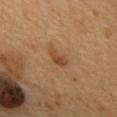follow-up = no biopsy performed (imaged during a skin exam)
illumination = cross-polarized illumination
patient = male, aged 53–57
site = the upper back
acquisition = total-body-photography crop, ~15 mm field of view
lesion diameter = ~3 mm (longest diameter)